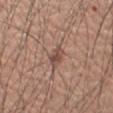Part of a total-body skin-imaging series; this lesion was reviewed on a skin check and was not flagged for biopsy. This is a white-light tile. A male patient in their 60s. The lesion is on the left forearm. About 3 mm across. Cropped from a whole-body photographic skin survey; the tile spans about 15 mm.